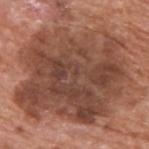- notes · imaged on a skin check; not biopsied
- TBP lesion metrics · an automated nevus-likeness rating near 0 out of 100 and a detector confidence of about 100 out of 100 that the crop contains a lesion
- diameter · ~12.5 mm (longest diameter)
- body site · the upper back
- patient · male, aged around 65
- imaging modality · ~15 mm crop, total-body skin-cancer survey